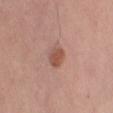Q: Is there a histopathology result?
A: no biopsy performed (imaged during a skin exam)
Q: What is the imaging modality?
A: ~15 mm tile from a whole-body skin photo
Q: What is the anatomic site?
A: the arm
Q: What are the patient's age and sex?
A: male, about 70 years old
Q: How was the tile lit?
A: white-light illumination
Q: Lesion size?
A: ~2.5 mm (longest diameter)